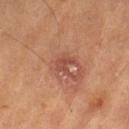Case summary:
* biopsy status: catalogued during a skin exam; not biopsied
* tile lighting: cross-polarized illumination
* acquisition: total-body-photography crop, ~15 mm field of view
* location: the leg
* size: ≈2.5 mm
* subject: male, aged 83 or over